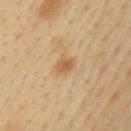Background:
The patient is a male about 40 years old. A region of skin cropped from a whole-body photographic capture, roughly 15 mm wide. An algorithmic analysis of the crop reported a nevus-likeness score of about 65/100 and lesion-presence confidence of about 100/100. Located on the left upper arm. Longest diameter approximately 2.5 mm.